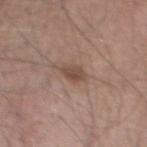Image and clinical context:
A male subject, aged 53–57. A lesion tile, about 15 mm wide, cut from a 3D total-body photograph. Captured under white-light illumination. About 2.5 mm across. The lesion is on the chest. The total-body-photography lesion software estimated a footprint of about 4.5 mm² and an outline eccentricity of about 0.6 (0 = round, 1 = elongated). The software also gave a border-irregularity rating of about 2.5/10 and a color-variation rating of about 2.5/10. It also reported a classifier nevus-likeness of about 75/100 and lesion-presence confidence of about 100/100.